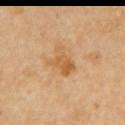Captured during whole-body skin photography for melanoma surveillance; the lesion was not biopsied. On the arm. The patient is a female about 65 years old. The tile uses cross-polarized illumination. This image is a 15 mm lesion crop taken from a total-body photograph. About 3.5 mm across. An algorithmic analysis of the crop reported an area of roughly 7 mm², an outline eccentricity of about 0.7 (0 = round, 1 = elongated), and two-axis asymmetry of about 0.45. And it measured a lesion color around L≈60 a*≈21 b*≈43 in CIELAB, about 9 CIELAB-L* units darker than the surrounding skin, and a lesion-to-skin contrast of about 7 (normalized; higher = more distinct). The software also gave a border-irregularity rating of about 5/10.